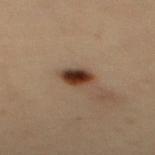{"biopsy_status": "not biopsied; imaged during a skin examination", "lighting": "cross-polarized", "site": "upper back", "patient": {"sex": "female", "age_approx": 30}, "image": {"source": "total-body photography crop", "field_of_view_mm": 15}, "lesion_size": {"long_diameter_mm_approx": 2.5}, "automated_metrics": {"eccentricity": 0.6, "shape_asymmetry": 0.15, "border_irregularity_0_10": 1.5, "color_variation_0_10": 5.5, "peripheral_color_asymmetry": 1.5}}